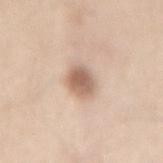biopsy_status: not biopsied; imaged during a skin examination
image:
  source: total-body photography crop
  field_of_view_mm: 15
automated_metrics:
  vs_skin_darker_L: 14.0
  vs_skin_contrast_norm: 8.5
site: lower back
patient:
  sex: male
  age_approx: 40
lighting: white-light
lesion_size:
  long_diameter_mm_approx: 3.5